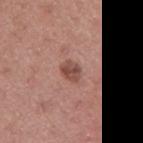| key | value |
|---|---|
| workup | catalogued during a skin exam; not biopsied |
| acquisition | ~15 mm crop, total-body skin-cancer survey |
| automated lesion analysis | border irregularity of about 2.5 on a 0–10 scale, internal color variation of about 3 on a 0–10 scale, and a peripheral color-asymmetry measure near 1; an automated nevus-likeness rating near 55 out of 100 |
| subject | male, aged 38 to 42 |
| lighting | white-light illumination |
| body site | the arm |
| lesion size | ≈2.5 mm |